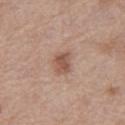Clinical impression:
Imaged during a routine full-body skin examination; the lesion was not biopsied and no histopathology is available.
Image and clinical context:
On the left thigh. This image is a 15 mm lesion crop taken from a total-body photograph. Automated image analysis of the tile measured a lesion–skin lightness drop of about 10 and a lesion-to-skin contrast of about 7 (normalized; higher = more distinct). It also reported a border-irregularity rating of about 2.5/10, internal color variation of about 2.5 on a 0–10 scale, and radial color variation of about 1. The software also gave an automated nevus-likeness rating near 65 out of 100 and lesion-presence confidence of about 100/100. Imaged with white-light lighting. The lesion's longest dimension is about 3 mm. A female patient approximately 40 years of age.